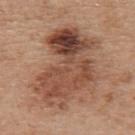The lesion was photographed on a routine skin check and not biopsied; there is no pathology result. On the upper back. This image is a 15 mm lesion crop taken from a total-body photograph. Captured under white-light illumination. A male patient approximately 70 years of age.